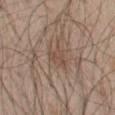Part of a total-body skin-imaging series; this lesion was reviewed on a skin check and was not flagged for biopsy. From the mid back. Captured under white-light illumination. A male subject in their 50s. This image is a 15 mm lesion crop taken from a total-body photograph.